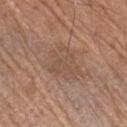notes=no biopsy performed (imaged during a skin exam)
imaging modality=15 mm crop, total-body photography
body site=the left forearm
patient=male, aged approximately 70